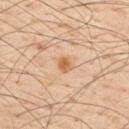The lesion was tiled from a total-body skin photograph and was not biopsied.